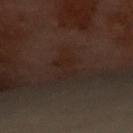Clinical impression: Captured during whole-body skin photography for melanoma surveillance; the lesion was not biopsied. Background: On the front of the torso. This is a cross-polarized tile. An algorithmic analysis of the crop reported an area of roughly 4.5 mm², an outline eccentricity of about 0.8 (0 = round, 1 = elongated), and a symmetry-axis asymmetry near 0.5. It also reported a nevus-likeness score of about 0/100 and a detector confidence of about 100 out of 100 that the crop contains a lesion. A female patient about 60 years old. The lesion's longest dimension is about 3.5 mm. A 15 mm close-up tile from a total-body photography series done for melanoma screening.